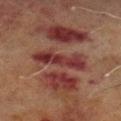biopsy_status: not biopsied; imaged during a skin examination
image:
  source: total-body photography crop
  field_of_view_mm: 15
lesion_size:
  long_diameter_mm_approx: 5.5
lighting: cross-polarized
site: left lower leg
automated_metrics:
  area_mm2_approx: 9.0
  eccentricity: 0.95
  shape_asymmetry: 0.3
  border_irregularity_0_10: 5.0
  peripheral_color_asymmetry: 1.5
  nevus_likeness_0_100: 0
  lesion_detection_confidence_0_100: 90
patient:
  sex: male
  age_approx: 70